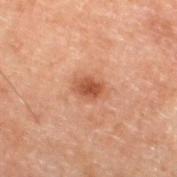| field | value |
|---|---|
| tile lighting | cross-polarized |
| subject | male, aged approximately 70 |
| site | the left lower leg |
| image source | ~15 mm crop, total-body skin-cancer survey |